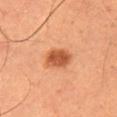Case summary:
– biopsy status · total-body-photography surveillance lesion; no biopsy
– image source · ~15 mm tile from a whole-body skin photo
– subject · male, aged 48 to 52
– anatomic site · the right thigh
– illumination · cross-polarized illumination
– lesion size · ~3.5 mm (longest diameter)